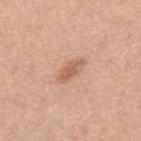The lesion is on the back.
Cropped from a whole-body photographic skin survey; the tile spans about 15 mm.
The recorded lesion diameter is about 3.5 mm.
This is a white-light tile.
The subject is a male aged around 45.